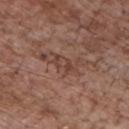Impression: Recorded during total-body skin imaging; not selected for excision or biopsy. Context: On the chest. The tile uses white-light illumination. A male subject, aged around 70. The lesion's longest dimension is about 3 mm. A lesion tile, about 15 mm wide, cut from a 3D total-body photograph. The total-body-photography lesion software estimated an outline eccentricity of about 0.85 (0 = round, 1 = elongated) and two-axis asymmetry of about 0.35. And it measured border irregularity of about 4.5 on a 0–10 scale, a color-variation rating of about 4/10, and radial color variation of about 1.5. The analysis additionally found lesion-presence confidence of about 55/100.